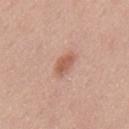{"biopsy_status": "not biopsied; imaged during a skin examination", "site": "mid back", "lighting": "white-light", "lesion_size": {"long_diameter_mm_approx": 3.0}, "automated_metrics": {"area_mm2_approx": 4.5, "shape_asymmetry": 0.2, "cielab_L": 58, "cielab_a": 23, "cielab_b": 29, "vs_skin_darker_L": 10.0, "vs_skin_contrast_norm": 7.0, "color_variation_0_10": 2.0, "peripheral_color_asymmetry": 1.0}, "patient": {"sex": "male", "age_approx": 45}, "image": {"source": "total-body photography crop", "field_of_view_mm": 15}}